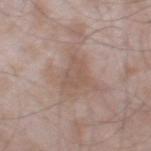<lesion>
<biopsy_status>not biopsied; imaged during a skin examination</biopsy_status>
<patient>
  <sex>male</sex>
  <age_approx>45</age_approx>
</patient>
<site>leg</site>
<image>
  <source>total-body photography crop</source>
  <field_of_view_mm>15</field_of_view_mm>
</image>
</lesion>